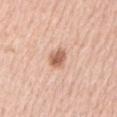Findings:
* workup — catalogued during a skin exam; not biopsied
* automated metrics — a footprint of about 5 mm² and two-axis asymmetry of about 0.2; a lesion color around L≈62 a*≈22 b*≈32 in CIELAB and a normalized border contrast of about 8.5; a within-lesion color-variation index near 4/10; an automated nevus-likeness rating near 95 out of 100 and lesion-presence confidence of about 100/100
* lesion diameter — ~3 mm (longest diameter)
* anatomic site — the left upper arm
* subject — male, aged 53–57
* image source — total-body-photography crop, ~15 mm field of view
* lighting — white-light